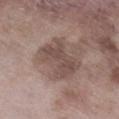Imaged during a routine full-body skin examination; the lesion was not biopsied and no histopathology is available. The tile uses white-light illumination. Approximately 5.5 mm at its widest. The lesion is located on the right lower leg. A male patient aged 58 to 62. A roughly 15 mm field-of-view crop from a total-body skin photograph. The total-body-photography lesion software estimated a mean CIELAB color near L≈48 a*≈15 b*≈21, a lesion–skin lightness drop of about 9, and a lesion-to-skin contrast of about 7 (normalized; higher = more distinct). It also reported a border-irregularity index near 3.5/10 and a color-variation rating of about 3/10.